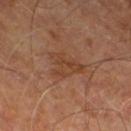Q: Is there a histopathology result?
A: no biopsy performed (imaged during a skin exam)
Q: What did automated image analysis measure?
A: a classifier nevus-likeness of about 0/100 and a lesion-detection confidence of about 100/100
Q: How was the tile lit?
A: cross-polarized
Q: Lesion location?
A: the leg
Q: Who is the patient?
A: approximately 65 years of age
Q: What is the imaging modality?
A: 15 mm crop, total-body photography